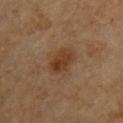Recorded during total-body skin imaging; not selected for excision or biopsy.
This is a cross-polarized tile.
Located on the upper back.
A lesion tile, about 15 mm wide, cut from a 3D total-body photograph.
The patient is a male aged around 85 or older.
The recorded lesion diameter is about 4 mm.